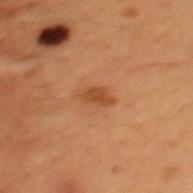Q: Is there a histopathology result?
A: no biopsy performed (imaged during a skin exam)
Q: What kind of image is this?
A: ~15 mm tile from a whole-body skin photo
Q: Automated lesion metrics?
A: an area of roughly 3.5 mm², an eccentricity of roughly 0.85, and a symmetry-axis asymmetry near 0.25; a lesion color around L≈38 a*≈21 b*≈32 in CIELAB and about 8 CIELAB-L* units darker than the surrounding skin
Q: What are the patient's age and sex?
A: male, aged 48 to 52
Q: What is the anatomic site?
A: the mid back